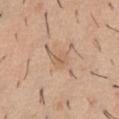Imaged during a routine full-body skin examination; the lesion was not biopsied and no histopathology is available. Imaged with white-light lighting. A close-up tile cropped from a whole-body skin photograph, about 15 mm across. Measured at roughly 3 mm in maximum diameter. The lesion is located on the chest. An algorithmic analysis of the crop reported a mean CIELAB color near L≈61 a*≈18 b*≈33. It also reported internal color variation of about 3 on a 0–10 scale and peripheral color asymmetry of about 1.5. The analysis additionally found a classifier nevus-likeness of about 0/100. A male subject, aged 38 to 42.